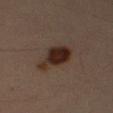The lesion was tiled from a total-body skin photograph and was not biopsied.
From the right upper arm.
A male subject, aged 33–37.
Cropped from a total-body skin-imaging series; the visible field is about 15 mm.